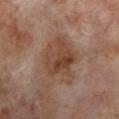The lesion was tiled from a total-body skin photograph and was not biopsied.
This is a cross-polarized tile.
Cropped from a whole-body photographic skin survey; the tile spans about 15 mm.
The patient is a male aged 68–72.
The total-body-photography lesion software estimated an average lesion color of about L≈42 a*≈19 b*≈28 (CIELAB), a lesion–skin lightness drop of about 9, and a lesion-to-skin contrast of about 8 (normalized; higher = more distinct). It also reported border irregularity of about 4 on a 0–10 scale, a color-variation rating of about 6/10, and radial color variation of about 2. And it measured a nevus-likeness score of about 10/100 and a detector confidence of about 100 out of 100 that the crop contains a lesion.
The lesion is located on the left lower leg.